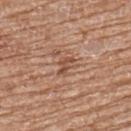A female subject aged approximately 70.
Located on the upper back.
The total-body-photography lesion software estimated a border-irregularity rating of about 5/10, internal color variation of about 1 on a 0–10 scale, and peripheral color asymmetry of about 0.5.
A region of skin cropped from a whole-body photographic capture, roughly 15 mm wide.